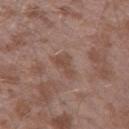Q: Was this lesion biopsied?
A: no biopsy performed (imaged during a skin exam)